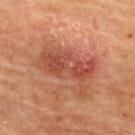Longest diameter approximately 6.5 mm. A region of skin cropped from a whole-body photographic capture, roughly 15 mm wide. A female subject aged around 80. From the back. The tile uses cross-polarized illumination.